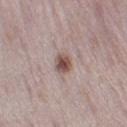notes: total-body-photography surveillance lesion; no biopsy
automated lesion analysis: a lesion area of about 4.5 mm² and an eccentricity of roughly 0.7; a classifier nevus-likeness of about 95/100 and a detector confidence of about 100 out of 100 that the crop contains a lesion
tile lighting: white-light illumination
lesion size: about 3 mm
acquisition: ~15 mm crop, total-body skin-cancer survey
patient: female, aged 28 to 32
anatomic site: the right thigh